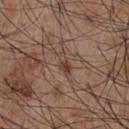<lesion>
<biopsy_status>not biopsied; imaged during a skin examination</biopsy_status>
<image>
  <source>total-body photography crop</source>
  <field_of_view_mm>15</field_of_view_mm>
</image>
<site>chest</site>
<lesion_size>
  <long_diameter_mm_approx>3.0</long_diameter_mm_approx>
</lesion_size>
<lighting>white-light</lighting>
<patient>
  <sex>male</sex>
  <age_approx>55</age_approx>
</patient>
</lesion>